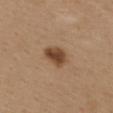<case>
<biopsy_status>not biopsied; imaged during a skin examination</biopsy_status>
<image>
  <source>total-body photography crop</source>
  <field_of_view_mm>15</field_of_view_mm>
</image>
<automated_metrics>
  <area_mm2_approx>6.5</area_mm2_approx>
  <eccentricity>0.75</eccentricity>
</automated_metrics>
<lesion_size>
  <long_diameter_mm_approx>3.5</long_diameter_mm_approx>
</lesion_size>
<patient>
  <sex>female</sex>
  <age_approx>40</age_approx>
</patient>
<lighting>white-light</lighting>
<site>back</site>
</case>